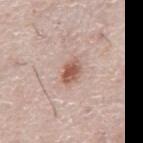workup = total-body-photography surveillance lesion; no biopsy
TBP lesion metrics = a footprint of about 5.5 mm²; roughly 13 lightness units darker than nearby skin and a normalized lesion–skin contrast near 9; a border-irregularity rating of about 2.5/10, a color-variation rating of about 3.5/10, and a peripheral color-asymmetry measure near 1
patient = male, aged 73 to 77
site = the abdomen
image source = 15 mm crop, total-body photography
lighting = white-light